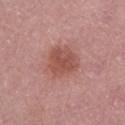Impression:
The lesion was tiled from a total-body skin photograph and was not biopsied.
Image and clinical context:
A 15 mm close-up tile from a total-body photography series done for melanoma screening. A female patient aged 63–67. Captured under white-light illumination. Measured at roughly 4 mm in maximum diameter. An algorithmic analysis of the crop reported an average lesion color of about L≈52 a*≈26 b*≈25 (CIELAB) and a lesion–skin lightness drop of about 9. The software also gave a border-irregularity rating of about 2/10 and radial color variation of about 1. Located on the leg.